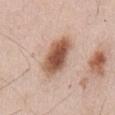From the abdomen.
A close-up tile cropped from a whole-body skin photograph, about 15 mm across.
Imaged with white-light lighting.
A male subject, aged 53 to 57.
Approximately 5.5 mm at its widest.